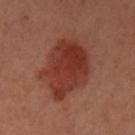Clinical impression:
Imaged during a routine full-body skin examination; the lesion was not biopsied and no histopathology is available.
Context:
A female patient aged 28–32. The recorded lesion diameter is about 7 mm. A close-up tile cropped from a whole-body skin photograph, about 15 mm across. This is a cross-polarized tile. Located on the left upper arm.